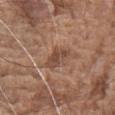Assessment:
The lesion was photographed on a routine skin check and not biopsied; there is no pathology result.
Image and clinical context:
The subject is a male roughly 75 years of age. A 15 mm crop from a total-body photograph taken for skin-cancer surveillance. The lesion is located on the front of the torso. An algorithmic analysis of the crop reported a lesion–skin lightness drop of about 9 and a normalized border contrast of about 7. The software also gave a border-irregularity rating of about 3/10, internal color variation of about 3.5 on a 0–10 scale, and peripheral color asymmetry of about 1. And it measured an automated nevus-likeness rating near 0 out of 100 and lesion-presence confidence of about 100/100.